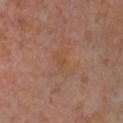<case>
  <biopsy_status>not biopsied; imaged during a skin examination</biopsy_status>
  <patient>
    <sex>female</sex>
    <age_approx>60</age_approx>
  </patient>
  <lighting>cross-polarized</lighting>
  <lesion_size>
    <long_diameter_mm_approx>3.5</long_diameter_mm_approx>
  </lesion_size>
  <automated_metrics>
    <border_irregularity_0_10>5.5</border_irregularity_0_10>
    <color_variation_0_10>1.5</color_variation_0_10>
    <peripheral_color_asymmetry>0.5</peripheral_color_asymmetry>
  </automated_metrics>
  <site>chest</site>
  <image>
    <source>total-body photography crop</source>
    <field_of_view_mm>15</field_of_view_mm>
  </image>
</case>